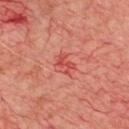The lesion was tiled from a total-body skin photograph and was not biopsied. About 2.5 mm across. A male patient, in their mid-60s. The lesion is located on the chest. Cropped from a whole-body photographic skin survey; the tile spans about 15 mm. Imaged with cross-polarized lighting.